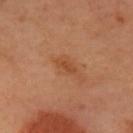| field | value |
|---|---|
| lesion diameter | about 2.5 mm |
| acquisition | total-body-photography crop, ~15 mm field of view |
| subject | female, aged 58–62 |
| anatomic site | the head or neck |
| lighting | cross-polarized |